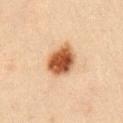Part of a total-body skin-imaging series; this lesion was reviewed on a skin check and was not flagged for biopsy.
A male patient aged 33 to 37.
Cropped from a total-body skin-imaging series; the visible field is about 15 mm.
On the right upper arm.